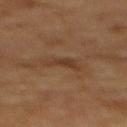workup: total-body-photography surveillance lesion; no biopsy.